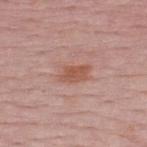Assessment:
The lesion was tiled from a total-body skin photograph and was not biopsied.
Background:
The recorded lesion diameter is about 3.5 mm. Automated image analysis of the tile measured a shape eccentricity near 0.8 and a shape-asymmetry score of about 0.25 (0 = symmetric). The software also gave a lesion color around L≈55 a*≈23 b*≈28 in CIELAB, about 8 CIELAB-L* units darker than the surrounding skin, and a normalized border contrast of about 7. The software also gave border irregularity of about 3 on a 0–10 scale, a within-lesion color-variation index near 2.5/10, and radial color variation of about 0.5. It also reported an automated nevus-likeness rating near 30 out of 100 and a lesion-detection confidence of about 100/100. Captured under white-light illumination. From the upper back. A 15 mm close-up extracted from a 3D total-body photography capture. A male subject aged approximately 50.